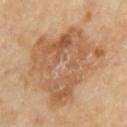  site: right upper arm
  image:
    source: total-body photography crop
    field_of_view_mm: 15
  patient:
    sex: male
    age_approx: 70